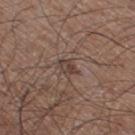– subject · male, approximately 60 years of age
– size · about 2.5 mm
– tile lighting · white-light illumination
– automated metrics · a footprint of about 4 mm² and a shape eccentricity near 0.75; a border-irregularity rating of about 2.5/10 and internal color variation of about 3 on a 0–10 scale; an automated nevus-likeness rating near 0 out of 100 and a detector confidence of about 70 out of 100 that the crop contains a lesion
– site · the right thigh
– imaging modality · ~15 mm tile from a whole-body skin photo